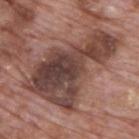Case summary:
- notes · no biopsy performed (imaged during a skin exam)
- image-analysis metrics · a footprint of about 46 mm², an eccentricity of roughly 0.85, and a shape-asymmetry score of about 0.45 (0 = symmetric); a lesion–skin lightness drop of about 14; internal color variation of about 7.5 on a 0–10 scale and a peripheral color-asymmetry measure near 2.5
- imaging modality · total-body-photography crop, ~15 mm field of view
- tile lighting · white-light illumination
- patient · male, in their 70s
- lesion size · ≈9.5 mm
- body site · the back